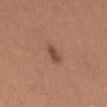Impression: The lesion was tiled from a total-body skin photograph and was not biopsied. Clinical summary: A female patient approximately 25 years of age. A lesion tile, about 15 mm wide, cut from a 3D total-body photograph. From the front of the torso. The tile uses white-light illumination. Automated image analysis of the tile measured a footprint of about 3 mm², an outline eccentricity of about 0.85 (0 = round, 1 = elongated), and a shape-asymmetry score of about 0.2 (0 = symmetric). The analysis additionally found a border-irregularity rating of about 2/10 and a peripheral color-asymmetry measure near 0.